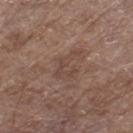Q: Was this lesion biopsied?
A: imaged on a skin check; not biopsied
Q: Illumination type?
A: white-light illumination
Q: What kind of image is this?
A: total-body-photography crop, ~15 mm field of view
Q: Who is the patient?
A: male, aged 68–72
Q: Where on the body is the lesion?
A: the leg
Q: Automated lesion metrics?
A: an outline eccentricity of about 0.85 (0 = round, 1 = elongated) and two-axis asymmetry of about 0.6
Q: How large is the lesion?
A: about 4 mm